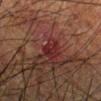No biopsy was performed on this lesion — it was imaged during a full skin examination and was not determined to be concerning. The patient is a male aged 58–62. Captured under cross-polarized illumination. The lesion is located on the left forearm. The total-body-photography lesion software estimated a mean CIELAB color near L≈20 a*≈22 b*≈18, roughly 6 lightness units darker than nearby skin, and a lesion-to-skin contrast of about 7 (normalized; higher = more distinct). The software also gave a border-irregularity rating of about 3.5/10, a within-lesion color-variation index near 2.5/10, and a peripheral color-asymmetry measure near 1. Longest diameter approximately 3 mm. Cropped from a whole-body photographic skin survey; the tile spans about 15 mm.